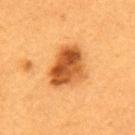Assessment:
This lesion was catalogued during total-body skin photography and was not selected for biopsy.
Image and clinical context:
The lesion is on the back. The tile uses cross-polarized illumination. Automated tile analysis of the lesion measured an area of roughly 14 mm², an outline eccentricity of about 0.7 (0 = round, 1 = elongated), and a symmetry-axis asymmetry near 0.25. The analysis additionally found a within-lesion color-variation index near 7.5/10 and a peripheral color-asymmetry measure near 2.5. The patient is a female roughly 35 years of age. Longest diameter approximately 5.5 mm. Cropped from a total-body skin-imaging series; the visible field is about 15 mm.